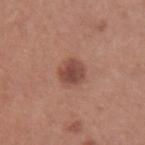The lesion was photographed on a routine skin check and not biopsied; there is no pathology result. The tile uses white-light illumination. A female subject, about 30 years old. The lesion is located on the left upper arm. The lesion's longest dimension is about 3 mm. A roughly 15 mm field-of-view crop from a total-body skin photograph.